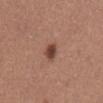No biopsy was performed on this lesion — it was imaged during a full skin examination and was not determined to be concerning. Located on the abdomen. About 2.5 mm across. Imaged with white-light lighting. A female patient in their 50s. A region of skin cropped from a whole-body photographic capture, roughly 15 mm wide. Automated image analysis of the tile measured an eccentricity of roughly 0.65 and a shape-asymmetry score of about 0.2 (0 = symmetric). It also reported a mean CIELAB color near L≈44 a*≈22 b*≈26 and a lesion-to-skin contrast of about 9.5 (normalized; higher = more distinct).